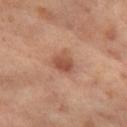<record>
<biopsy_status>not biopsied; imaged during a skin examination</biopsy_status>
<patient>
  <sex>female</sex>
  <age_approx>55</age_approx>
</patient>
<site>left thigh</site>
<image>
  <source>total-body photography crop</source>
  <field_of_view_mm>15</field_of_view_mm>
</image>
</record>